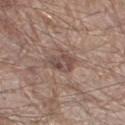This lesion was catalogued during total-body skin photography and was not selected for biopsy. A male patient aged around 80. Cropped from a total-body skin-imaging series; the visible field is about 15 mm. The lesion is on the right thigh.